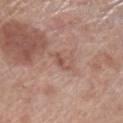{"biopsy_status": "not biopsied; imaged during a skin examination", "automated_metrics": {"area_mm2_approx": 2.5, "shape_asymmetry": 0.35, "color_variation_0_10": 0.0, "peripheral_color_asymmetry": 0.0}, "site": "left lower leg", "image": {"source": "total-body photography crop", "field_of_view_mm": 15}, "lesion_size": {"long_diameter_mm_approx": 2.5}, "patient": {"sex": "male", "age_approx": 70}}